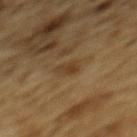The lesion was tiled from a total-body skin photograph and was not biopsied. The lesion is on the upper back. A male patient roughly 85 years of age or older. A 15 mm crop from a total-body photograph taken for skin-cancer surveillance.